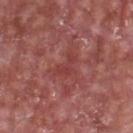Part of a total-body skin-imaging series; this lesion was reviewed on a skin check and was not flagged for biopsy. Automated image analysis of the tile measured a lesion area of about 4.5 mm² and a shape eccentricity near 0.85. The software also gave a mean CIELAB color near L≈41 a*≈31 b*≈24 and a lesion–skin lightness drop of about 6. The analysis additionally found border irregularity of about 7 on a 0–10 scale, a within-lesion color-variation index near 0.5/10, and a peripheral color-asymmetry measure near 0. The software also gave an automated nevus-likeness rating near 0 out of 100 and a lesion-detection confidence of about 90/100. The lesion's longest dimension is about 3.5 mm. The lesion is located on the chest. The tile uses white-light illumination. A lesion tile, about 15 mm wide, cut from a 3D total-body photograph. A male patient, in their mid-60s.